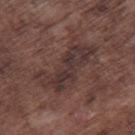Q: Was this lesion biopsied?
A: catalogued during a skin exam; not biopsied